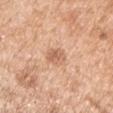notes: imaged on a skin check; not biopsied | anatomic site: the arm | subject: male, aged around 55 | diameter: ~2.5 mm (longest diameter) | imaging modality: 15 mm crop, total-body photography.